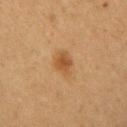Part of a total-body skin-imaging series; this lesion was reviewed on a skin check and was not flagged for biopsy.
The lesion's longest dimension is about 3 mm.
A 15 mm crop from a total-body photograph taken for skin-cancer surveillance.
Automated image analysis of the tile measured roughly 8 lightness units darker than nearby skin and a normalized lesion–skin contrast near 7. The analysis additionally found a classifier nevus-likeness of about 90/100 and a detector confidence of about 100 out of 100 that the crop contains a lesion.
The subject is a female aged 38 to 42.
The tile uses cross-polarized illumination.
Located on the right upper arm.